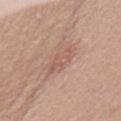biopsy_status: not biopsied; imaged during a skin examination
image:
  source: total-body photography crop
  field_of_view_mm: 15
automated_metrics:
  vs_skin_darker_L: 7.0
  vs_skin_contrast_norm: 5.0
  nevus_likeness_0_100: 0
  lesion_detection_confidence_0_100: 100
site: chest
patient:
  sex: female
  age_approx: 55
lighting: white-light